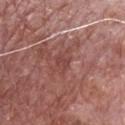The lesion was tiled from a total-body skin photograph and was not biopsied.
Longest diameter approximately 3.5 mm.
A male patient, in their 70s.
On the chest.
A close-up tile cropped from a whole-body skin photograph, about 15 mm across.